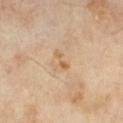This lesion was catalogued during total-body skin photography and was not selected for biopsy. The lesion is on the left lower leg. This is a cross-polarized tile. A 15 mm close-up extracted from a 3D total-body photography capture. The subject is a female aged approximately 70. The total-body-photography lesion software estimated lesion-presence confidence of about 100/100. Approximately 2.5 mm at its widest.